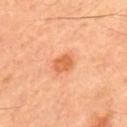Imaged during a routine full-body skin examination; the lesion was not biopsied and no histopathology is available. Automated tile analysis of the lesion measured a border-irregularity rating of about 1.5/10, internal color variation of about 2.5 on a 0–10 scale, and radial color variation of about 1. The analysis additionally found a nevus-likeness score of about 95/100 and lesion-presence confidence of about 100/100. A male patient aged 48–52. The lesion is located on the back. Longest diameter approximately 2.5 mm. This is a cross-polarized tile. A region of skin cropped from a whole-body photographic capture, roughly 15 mm wide.